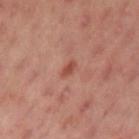follow-up: no biopsy performed (imaged during a skin exam) | subject: female, aged approximately 40 | body site: the left upper arm | acquisition: ~15 mm tile from a whole-body skin photo.